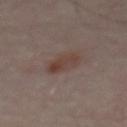follow-up = imaged on a skin check; not biopsied, patient = aged 53–57, image-analysis metrics = a footprint of about 6.5 mm², illumination = cross-polarized, acquisition = ~15 mm tile from a whole-body skin photo, lesion size = ≈3.5 mm, anatomic site = the abdomen.